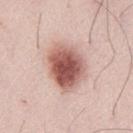Part of a total-body skin-imaging series; this lesion was reviewed on a skin check and was not flagged for biopsy. The patient is a male aged around 35. Imaged with white-light lighting. A close-up tile cropped from a whole-body skin photograph, about 15 mm across. The lesion is on the left thigh. Approximately 5.5 mm at its widest.